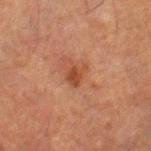Part of a total-body skin-imaging series; this lesion was reviewed on a skin check and was not flagged for biopsy. A 15 mm close-up extracted from a 3D total-body photography capture. The lesion is on the left lower leg. A male subject aged approximately 65.